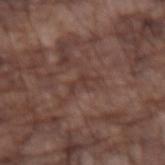Assessment: This lesion was catalogued during total-body skin photography and was not selected for biopsy. Context: A close-up tile cropped from a whole-body skin photograph, about 15 mm across. This is a white-light tile. The lesion's longest dimension is about 3 mm. From the left forearm. The subject is a male in their mid-70s.